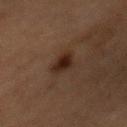Clinical impression: The lesion was photographed on a routine skin check and not biopsied; there is no pathology result. Background: An algorithmic analysis of the crop reported a lesion area of about 6 mm² and an eccentricity of roughly 0.95. The software also gave an average lesion color of about L≈20 a*≈14 b*≈19 (CIELAB). The software also gave radial color variation of about 1.5. The subject is a female approximately 60 years of age. A roughly 15 mm field-of-view crop from a total-body skin photograph. Longest diameter approximately 4.5 mm. The lesion is on the mid back.